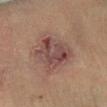Case summary:
• workup · imaged on a skin check; not biopsied
• body site · the right lower leg
• lesion size · ~5.5 mm (longest diameter)
• automated lesion analysis · a lesion color around L≈40 a*≈18 b*≈20 in CIELAB, a lesion–skin lightness drop of about 9, and a lesion-to-skin contrast of about 8 (normalized; higher = more distinct); a border-irregularity index near 2.5/10 and a color-variation rating of about 6.5/10; a lesion-detection confidence of about 100/100
• subject · male, about 65 years old
• acquisition · 15 mm crop, total-body photography
• illumination · cross-polarized